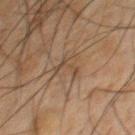Case summary:
– follow-up: no biopsy performed (imaged during a skin exam)
– tile lighting: cross-polarized
– diameter: ~3.5 mm (longest diameter)
– automated metrics: an area of roughly 3.5 mm² and a shape eccentricity near 0.9
– acquisition: ~15 mm tile from a whole-body skin photo
– subject: male, aged 58 to 62
– body site: the chest